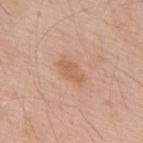<lesion>
<biopsy_status>not biopsied; imaged during a skin examination</biopsy_status>
<lesion_size>
  <long_diameter_mm_approx>3.5</long_diameter_mm_approx>
</lesion_size>
<patient>
  <sex>male</sex>
  <age_approx>55</age_approx>
</patient>
<image>
  <source>total-body photography crop</source>
  <field_of_view_mm>15</field_of_view_mm>
</image>
<lighting>white-light</lighting>
<site>upper back</site>
<automated_metrics>
  <cielab_L>60</cielab_L>
  <cielab_a>22</cielab_a>
  <cielab_b>34</cielab_b>
  <vs_skin_darker_L>7.0</vs_skin_darker_L>
  <vs_skin_contrast_norm>6.0</vs_skin_contrast_norm>
  <color_variation_0_10>2.0</color_variation_0_10>
  <peripheral_color_asymmetry>0.5</peripheral_color_asymmetry>
  <nevus_likeness_0_100>15</nevus_likeness_0_100>
  <lesion_detection_confidence_0_100>100</lesion_detection_confidence_0_100>
</automated_metrics>
</lesion>